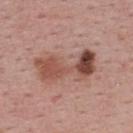Part of a total-body skin-imaging series; this lesion was reviewed on a skin check and was not flagged for biopsy. A 15 mm crop from a total-body photograph taken for skin-cancer surveillance. Automated tile analysis of the lesion measured border irregularity of about 6 on a 0–10 scale, internal color variation of about 9.5 on a 0–10 scale, and a peripheral color-asymmetry measure near 3.5. A female patient, aged 53 to 57. The lesion's longest dimension is about 7 mm. From the back. Captured under white-light illumination.